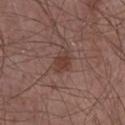This lesion was catalogued during total-body skin photography and was not selected for biopsy. The total-body-photography lesion software estimated a lesion area of about 4 mm², an eccentricity of roughly 0.7, and a shape-asymmetry score of about 0.2 (0 = symmetric). The software also gave a border-irregularity index near 2/10, a color-variation rating of about 2.5/10, and peripheral color asymmetry of about 1. And it measured an automated nevus-likeness rating near 60 out of 100 and a detector confidence of about 100 out of 100 that the crop contains a lesion. Cropped from a whole-body photographic skin survey; the tile spans about 15 mm. Imaged with white-light lighting. The lesion is located on the chest. A male patient, aged around 60.